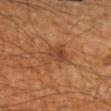Imaged during a routine full-body skin examination; the lesion was not biopsied and no histopathology is available. A male subject in their 60s. The total-body-photography lesion software estimated a mean CIELAB color near L≈43 a*≈23 b*≈34, about 8 CIELAB-L* units darker than the surrounding skin, and a normalized lesion–skin contrast near 6.5. Measured at roughly 4 mm in maximum diameter. A close-up tile cropped from a whole-body skin photograph, about 15 mm across. This is a cross-polarized tile. On the left upper arm.